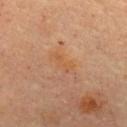| feature | finding |
|---|---|
| follow-up | catalogued during a skin exam; not biopsied |
| image source | total-body-photography crop, ~15 mm field of view |
| body site | the chest |
| subject | female, about 60 years old |
| lesion size | ~3.5 mm (longest diameter) |